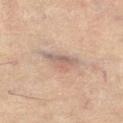Q: Was this lesion biopsied?
A: no biopsy performed (imaged during a skin exam)
Q: What did automated image analysis measure?
A: a lesion area of about 6 mm² and an eccentricity of roughly 0.85; an average lesion color of about L≈54 a*≈15 b*≈22 (CIELAB), a lesion–skin lightness drop of about 8, and a normalized border contrast of about 6.5
Q: Where on the body is the lesion?
A: the right thigh
Q: What is the imaging modality?
A: total-body-photography crop, ~15 mm field of view
Q: Illumination type?
A: cross-polarized illumination
Q: Who is the patient?
A: male, roughly 60 years of age
Q: Lesion size?
A: about 4 mm